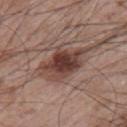Impression:
No biopsy was performed on this lesion — it was imaged during a full skin examination and was not determined to be concerning.
Clinical summary:
On the left upper arm. Cropped from a total-body skin-imaging series; the visible field is about 15 mm. This is a white-light tile. About 5.5 mm across. A male patient in their mid-60s. An algorithmic analysis of the crop reported a lesion-to-skin contrast of about 10.5 (normalized; higher = more distinct). The software also gave a classifier nevus-likeness of about 80/100.